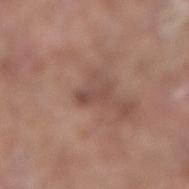Part of a total-body skin-imaging series; this lesion was reviewed on a skin check and was not flagged for biopsy. The subject is a male roughly 60 years of age. The recorded lesion diameter is about 3 mm. A roughly 15 mm field-of-view crop from a total-body skin photograph. Captured under white-light illumination. The lesion is located on the right forearm.